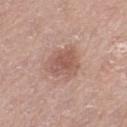Captured during whole-body skin photography for melanoma surveillance; the lesion was not biopsied.